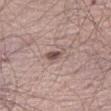From the abdomen. The patient is a male in their mid-60s. This is a white-light tile. The lesion-visualizer software estimated a footprint of about 3.5 mm² and an eccentricity of roughly 0.8. And it measured a border-irregularity index near 4.5/10, a within-lesion color-variation index near 3/10, and peripheral color asymmetry of about 1. A 15 mm close-up tile from a total-body photography series done for melanoma screening.